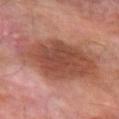- location — the left forearm
- size — ≈9.5 mm
- subject — male, aged approximately 70
- image source — ~15 mm crop, total-body skin-cancer survey
- lighting — cross-polarized
- TBP lesion metrics — a lesion area of about 42 mm², a shape eccentricity near 0.8, and two-axis asymmetry of about 0.2; a classifier nevus-likeness of about 25/100 and a lesion-detection confidence of about 100/100